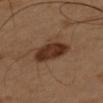Case summary:
– workup — imaged on a skin check; not biopsied
– tile lighting — cross-polarized illumination
– imaging modality — ~15 mm tile from a whole-body skin photo
– subject — male, aged 48–52
– body site — the arm